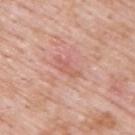Acquisition and patient details: The subject is a male aged 53–57. A 15 mm close-up tile from a total-body photography series done for melanoma screening. From the upper back. Approximately 3.5 mm at its widest. The tile uses white-light illumination. An algorithmic analysis of the crop reported a footprint of about 3.5 mm² and a shape-asymmetry score of about 0.4 (0 = symmetric). The analysis additionally found an average lesion color of about L≈60 a*≈26 b*≈28 (CIELAB) and a normalized lesion–skin contrast near 4.5. The analysis additionally found a within-lesion color-variation index near 0.5/10 and peripheral color asymmetry of about 0. And it measured an automated nevus-likeness rating near 0 out of 100.